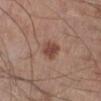Recorded during total-body skin imaging; not selected for excision or biopsy. A roughly 15 mm field-of-view crop from a total-body skin photograph. The lesion is on the right lower leg. Imaged with white-light lighting. A male subject, in their mid- to late 50s. Longest diameter approximately 2.5 mm.